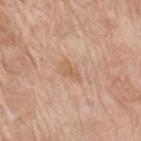Q: Was this lesion biopsied?
A: imaged on a skin check; not biopsied
Q: How was the tile lit?
A: white-light illumination
Q: What kind of image is this?
A: ~15 mm crop, total-body skin-cancer survey
Q: How large is the lesion?
A: ≈2.5 mm
Q: What is the anatomic site?
A: the right upper arm
Q: Who is the patient?
A: female, about 75 years old
Q: Automated lesion metrics?
A: an area of roughly 3 mm² and a symmetry-axis asymmetry near 0.35; a mean CIELAB color near L≈61 a*≈20 b*≈33, roughly 7 lightness units darker than nearby skin, and a normalized border contrast of about 5.5